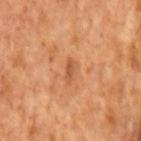Notes:
* notes: catalogued during a skin exam; not biopsied
* image source: ~15 mm tile from a whole-body skin photo
* patient: male, aged approximately 65
* illumination: cross-polarized